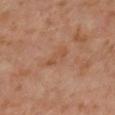The lesion was tiled from a total-body skin photograph and was not biopsied. A male subject, aged 58 to 62. Automated tile analysis of the lesion measured a lesion area of about 4 mm², a shape eccentricity near 0.9, and a shape-asymmetry score of about 0.4 (0 = symmetric). The analysis additionally found a normalized border contrast of about 5. The lesion is located on the back. Cropped from a total-body skin-imaging series; the visible field is about 15 mm. Longest diameter approximately 3.5 mm. Captured under cross-polarized illumination.